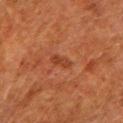Recorded during total-body skin imaging; not selected for excision or biopsy. The subject is a male aged 78–82. The lesion-visualizer software estimated a lesion–skin lightness drop of about 6 and a normalized lesion–skin contrast near 6. The analysis additionally found a nevus-likeness score of about 0/100 and a detector confidence of about 100 out of 100 that the crop contains a lesion. Captured under cross-polarized illumination. From the left lower leg. Longest diameter approximately 3 mm. A roughly 15 mm field-of-view crop from a total-body skin photograph.